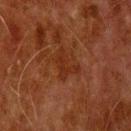  biopsy_status: not biopsied; imaged during a skin examination
  site: head or neck
  lighting: cross-polarized
  patient:
    sex: male
    age_approx: 80
  automated_metrics:
    area_mm2_approx: 6.0
    shape_asymmetry: 0.45
    cielab_L: 23
    cielab_a: 21
    cielab_b: 27
    vs_skin_darker_L: 5.0
    vs_skin_contrast_norm: 5.5
    color_variation_0_10: 1.0
    peripheral_color_asymmetry: 0.5
    lesion_detection_confidence_0_100: 100
  image:
    source: total-body photography crop
    field_of_view_mm: 15